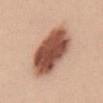Q: Is there a histopathology result?
A: total-body-photography surveillance lesion; no biopsy
Q: How was this image acquired?
A: total-body-photography crop, ~15 mm field of view
Q: Illumination type?
A: white-light illumination
Q: What did automated image analysis measure?
A: a lesion area of about 28 mm², a shape eccentricity near 0.8, and a symmetry-axis asymmetry near 0.15; lesion-presence confidence of about 100/100
Q: Lesion location?
A: the chest
Q: What is the lesion's diameter?
A: about 8 mm
Q: Who is the patient?
A: female, in their mid-20s